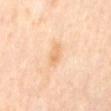follow-up = no biopsy performed (imaged during a skin exam); location = the mid back; automated lesion analysis = a border-irregularity rating of about 2/10, a within-lesion color-variation index near 2.5/10, and radial color variation of about 1; imaging modality = total-body-photography crop, ~15 mm field of view; lighting = cross-polarized; patient = female, approximately 50 years of age.